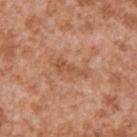Notes:
* workup · imaged on a skin check; not biopsied
* acquisition · 15 mm crop, total-body photography
* image-analysis metrics · an area of roughly 4.5 mm², a shape eccentricity near 0.95, and two-axis asymmetry of about 0.5; a nevus-likeness score of about 0/100 and a lesion-detection confidence of about 100/100
* subject · male, aged around 45
* location · the right upper arm